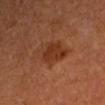No biopsy was performed on this lesion — it was imaged during a full skin examination and was not determined to be concerning. A female subject, approximately 35 years of age. Located on the left forearm. Cropped from a total-body skin-imaging series; the visible field is about 15 mm. This is a cross-polarized tile.